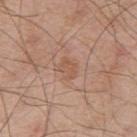Findings:
- workup — total-body-photography surveillance lesion; no biopsy
- patient — male, about 65 years old
- anatomic site — the right upper arm
- acquisition — total-body-photography crop, ~15 mm field of view